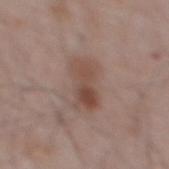Notes:
• follow-up: imaged on a skin check; not biopsied
• patient: male, aged 63 to 67
• illumination: white-light illumination
• acquisition: 15 mm crop, total-body photography
• automated metrics: a lesion color around L≈48 a*≈17 b*≈24 in CIELAB; a border-irregularity index near 2.5/10, internal color variation of about 6.5 on a 0–10 scale, and peripheral color asymmetry of about 2.5
• site: the mid back
• diameter: ≈4.5 mm